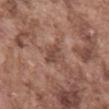follow-up = total-body-photography surveillance lesion; no biopsy | lesion diameter = ≈3 mm | illumination = white-light illumination | location = the back | image = 15 mm crop, total-body photography | subject = male, aged 73 to 77 | automated lesion analysis = a mean CIELAB color near L≈46 a*≈20 b*≈25 and a normalized border contrast of about 6.5; an automated nevus-likeness rating near 0 out of 100 and a detector confidence of about 100 out of 100 that the crop contains a lesion.